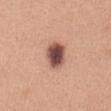Findings:
- workup · catalogued during a skin exam; not biopsied
- imaging modality · ~15 mm crop, total-body skin-cancer survey
- lesion diameter · about 4 mm
- patient · female, aged 28 to 32
- location · the front of the torso